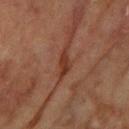Image and clinical context: Imaged with cross-polarized lighting. An algorithmic analysis of the crop reported an area of roughly 2 mm² and a shape-asymmetry score of about 0.45 (0 = symmetric). The lesion is on the left forearm. A roughly 15 mm field-of-view crop from a total-body skin photograph. A female subject, approximately 80 years of age.